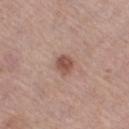No biopsy was performed on this lesion — it was imaged during a full skin examination and was not determined to be concerning. A 15 mm close-up extracted from a 3D total-body photography capture. This is a white-light tile. The lesion is located on the right thigh. Approximately 2.5 mm at its widest. A female subject, about 65 years old. Automated image analysis of the tile measured a footprint of about 4.5 mm², an eccentricity of roughly 0.55, and two-axis asymmetry of about 0.15. The analysis additionally found a lesion color around L≈51 a*≈22 b*≈25 in CIELAB, roughly 11 lightness units darker than nearby skin, and a lesion-to-skin contrast of about 8 (normalized; higher = more distinct).